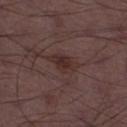Recorded during total-body skin imaging; not selected for excision or biopsy. The lesion's longest dimension is about 4 mm. A male patient aged approximately 50. Cropped from a whole-body photographic skin survey; the tile spans about 15 mm. The lesion is on the leg. The tile uses white-light illumination.